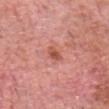{
  "biopsy_status": "not biopsied; imaged during a skin examination",
  "automated_metrics": {
    "area_mm2_approx": 3.0,
    "eccentricity": 0.85,
    "shape_asymmetry": 0.35,
    "cielab_L": 54,
    "cielab_a": 30,
    "cielab_b": 30,
    "vs_skin_darker_L": 10.0,
    "vs_skin_contrast_norm": 7.0,
    "border_irregularity_0_10": 3.0,
    "color_variation_0_10": 3.0,
    "nevus_likeness_0_100": 10,
    "lesion_detection_confidence_0_100": 100
  },
  "image": {
    "source": "total-body photography crop",
    "field_of_view_mm": 15
  },
  "site": "head or neck",
  "lighting": "white-light",
  "patient": {
    "sex": "male",
    "age_approx": 80
  }
}